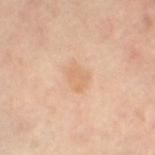Clinical impression: Imaged during a routine full-body skin examination; the lesion was not biopsied and no histopathology is available. Background: The patient is a female aged approximately 50. Longest diameter approximately 3 mm. Cropped from a whole-body photographic skin survey; the tile spans about 15 mm. The lesion is located on the left thigh.